A close-up tile cropped from a whole-body skin photograph, about 15 mm across · the patient is a male aged approximately 60 · located on the mid back.
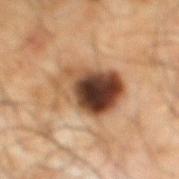Captured under cross-polarized illumination.
The lesion's longest dimension is about 7 mm.
Histopathological examination showed a seborrheic keratosis.